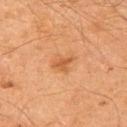Captured during whole-body skin photography for melanoma surveillance; the lesion was not biopsied.
This is a cross-polarized tile.
Approximately 3 mm at its widest.
The lesion is on the left upper arm.
A 15 mm close-up tile from a total-body photography series done for melanoma screening.
The subject is a male aged approximately 65.